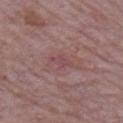The lesion's longest dimension is about 3 mm.
The tile uses white-light illumination.
The subject is a male in their mid-60s.
Cropped from a total-body skin-imaging series; the visible field is about 15 mm.
Located on the right upper arm.